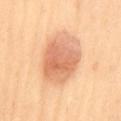Located on the mid back. Imaged with cross-polarized lighting. The patient is a female roughly 60 years of age. A 15 mm close-up extracted from a 3D total-body photography capture.